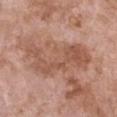About 8.5 mm across. The lesion is located on the chest. A female patient, aged 73–77. Automated image analysis of the tile measured a normalized lesion–skin contrast near 6.5. The software also gave a border-irregularity rating of about 8/10 and internal color variation of about 3.5 on a 0–10 scale. And it measured an automated nevus-likeness rating near 0 out of 100 and a detector confidence of about 95 out of 100 that the crop contains a lesion. Imaged with white-light lighting. A roughly 15 mm field-of-view crop from a total-body skin photograph.